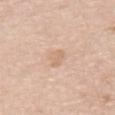• workup · total-body-photography surveillance lesion; no biopsy